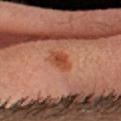Imaged during a routine full-body skin examination; the lesion was not biopsied and no histopathology is available.